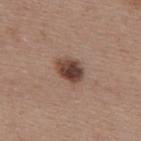Assessment: No biopsy was performed on this lesion — it was imaged during a full skin examination and was not determined to be concerning. Clinical summary: The lesion is on the back. This image is a 15 mm lesion crop taken from a total-body photograph. The patient is a female aged approximately 45. Captured under white-light illumination.